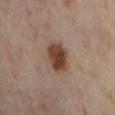The lesion was photographed on a routine skin check and not biopsied; there is no pathology result. About 4 mm across. The lesion is located on the left lower leg. A female subject, in their mid- to late 50s. A region of skin cropped from a whole-body photographic capture, roughly 15 mm wide. Imaged with cross-polarized lighting.